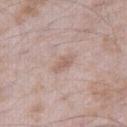Q: Is there a histopathology result?
A: total-body-photography surveillance lesion; no biopsy
Q: How was the tile lit?
A: white-light
Q: Automated lesion metrics?
A: a lesion area of about 3 mm²; a classifier nevus-likeness of about 0/100
Q: How was this image acquired?
A: ~15 mm tile from a whole-body skin photo
Q: What are the patient's age and sex?
A: male, in their mid- to late 40s
Q: Lesion location?
A: the right thigh
Q: How large is the lesion?
A: ~3 mm (longest diameter)